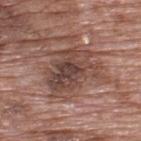The lesion was photographed on a routine skin check and not biopsied; there is no pathology result.
A male patient, about 70 years old.
Cropped from a total-body skin-imaging series; the visible field is about 15 mm.
Imaged with white-light lighting.
Approximately 6.5 mm at its widest.
Located on the upper back.